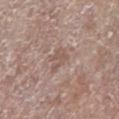Captured during whole-body skin photography for melanoma surveillance; the lesion was not biopsied. Captured under white-light illumination. A roughly 15 mm field-of-view crop from a total-body skin photograph. A female patient, aged 83 or older. The lesion is located on the left lower leg. Measured at roughly 3 mm in maximum diameter.